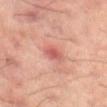site = the left thigh
acquisition = 15 mm crop, total-body photography
lighting = cross-polarized
subject = male, about 60 years old
lesion diameter = ~3 mm (longest diameter)
TBP lesion metrics = a lesion area of about 4 mm² and an eccentricity of roughly 0.85; an automated nevus-likeness rating near 0 out of 100